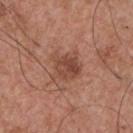biopsy status = total-body-photography surveillance lesion; no biopsy
body site = the chest
imaging modality = ~15 mm crop, total-body skin-cancer survey
patient = male, aged around 55